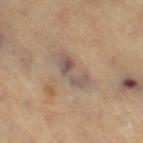Q: Is there a histopathology result?
A: no biopsy performed (imaged during a skin exam)
Q: Patient demographics?
A: female, in their 70s
Q: What is the lesion's diameter?
A: about 5 mm
Q: How was this image acquired?
A: 15 mm crop, total-body photography
Q: Lesion location?
A: the right thigh